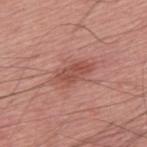Captured during whole-body skin photography for melanoma surveillance; the lesion was not biopsied.